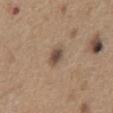Q: Is there a histopathology result?
A: catalogued during a skin exam; not biopsied
Q: How large is the lesion?
A: about 2.5 mm
Q: Lesion location?
A: the chest
Q: Who is the patient?
A: male, about 65 years old
Q: What kind of image is this?
A: ~15 mm crop, total-body skin-cancer survey